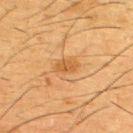Recorded during total-body skin imaging; not selected for excision or biopsy. A roughly 15 mm field-of-view crop from a total-body skin photograph. An algorithmic analysis of the crop reported a lesion color around L≈45 a*≈19 b*≈37 in CIELAB and about 7 CIELAB-L* units darker than the surrounding skin. It also reported a classifier nevus-likeness of about 50/100. A male subject aged around 35. From the upper back.